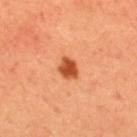Q: Was a biopsy performed?
A: no biopsy performed (imaged during a skin exam)
Q: What did automated image analysis measure?
A: an area of roughly 4.5 mm², an eccentricity of roughly 0.65, and two-axis asymmetry of about 0.2; a mean CIELAB color near L≈50 a*≈33 b*≈42 and about 16 CIELAB-L* units darker than the surrounding skin
Q: What is the imaging modality?
A: ~15 mm crop, total-body skin-cancer survey
Q: Patient demographics?
A: male, in their mid-60s
Q: What lighting was used for the tile?
A: cross-polarized
Q: What is the anatomic site?
A: the upper back